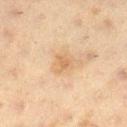Findings:
– follow-up — catalogued during a skin exam; not biopsied
– image — 15 mm crop, total-body photography
– TBP lesion metrics — an area of roughly 4.5 mm², an outline eccentricity of about 0.6 (0 = round, 1 = elongated), and a shape-asymmetry score of about 0.3 (0 = symmetric); a lesion color around L≈55 a*≈15 b*≈33 in CIELAB, roughly 6 lightness units darker than nearby skin, and a lesion-to-skin contrast of about 5.5 (normalized; higher = more distinct); a border-irregularity index near 3/10 and peripheral color asymmetry of about 1; a nevus-likeness score of about 0/100 and a detector confidence of about 100 out of 100 that the crop contains a lesion
– site — the right lower leg
– size — about 2.5 mm
– subject — female, about 40 years old